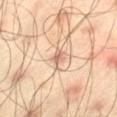Captured during whole-body skin photography for melanoma surveillance; the lesion was not biopsied. This is a cross-polarized tile. An algorithmic analysis of the crop reported a lesion area of about 3.5 mm² and two-axis asymmetry of about 0.25. The analysis additionally found a lesion color around L≈67 a*≈19 b*≈31 in CIELAB, a lesion–skin lightness drop of about 12, and a normalized lesion–skin contrast near 7. It also reported a border-irregularity index near 3/10, internal color variation of about 3.5 on a 0–10 scale, and radial color variation of about 1.5. A 15 mm close-up extracted from a 3D total-body photography capture. The patient is a male in their mid- to late 40s. From the leg.